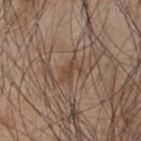notes — no biopsy performed (imaged during a skin exam)
subject — male, in their mid- to late 40s
anatomic site — the upper back
automated lesion analysis — a lesion–skin lightness drop of about 7 and a normalized lesion–skin contrast near 6; a border-irregularity index near 5.5/10 and radial color variation of about 0; a nevus-likeness score of about 0/100
lesion size — about 3 mm
image — 15 mm crop, total-body photography
tile lighting — white-light illumination